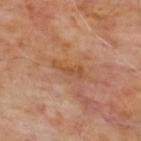| feature | finding |
|---|---|
| workup | catalogued during a skin exam; not biopsied |
| imaging modality | ~15 mm crop, total-body skin-cancer survey |
| body site | the upper back |
| subject | male, aged 63 to 67 |